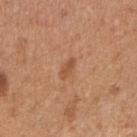Impression: Recorded during total-body skin imaging; not selected for excision or biopsy. Context: A roughly 15 mm field-of-view crop from a total-body skin photograph. Captured under white-light illumination. The lesion is on the arm. A female subject, roughly 30 years of age.